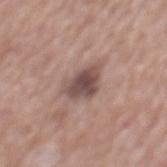Imaged during a routine full-body skin examination; the lesion was not biopsied and no histopathology is available. Imaged with white-light lighting. The total-body-photography lesion software estimated an average lesion color of about L≈48 a*≈18 b*≈20 (CIELAB) and a lesion-to-skin contrast of about 9.5 (normalized; higher = more distinct). And it measured a border-irregularity rating of about 3.5/10 and a color-variation rating of about 4/10. Cropped from a total-body skin-imaging series; the visible field is about 15 mm. The lesion is located on the mid back. A male patient, about 75 years old. The recorded lesion diameter is about 4.5 mm.